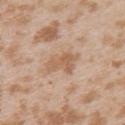The patient is a female about 25 years old. The tile uses white-light illumination. The lesion is located on the upper back. A roughly 15 mm field-of-view crop from a total-body skin photograph.